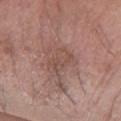• biopsy status: imaged on a skin check; not biopsied
• image source: ~15 mm tile from a whole-body skin photo
• patient: male, aged 58–62
• anatomic site: the right forearm
• lighting: white-light